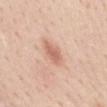Q: Was this lesion biopsied?
A: catalogued during a skin exam; not biopsied
Q: What lighting was used for the tile?
A: white-light
Q: Lesion size?
A: ~3.5 mm (longest diameter)
Q: How was this image acquired?
A: total-body-photography crop, ~15 mm field of view
Q: What did automated image analysis measure?
A: two-axis asymmetry of about 0.2; an average lesion color of about L≈64 a*≈23 b*≈30 (CIELAB) and a normalized border contrast of about 6.5; border irregularity of about 2 on a 0–10 scale
Q: Who is the patient?
A: female, in their mid- to late 40s
Q: Lesion location?
A: the mid back